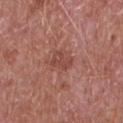| key | value |
|---|---|
| follow-up | imaged on a skin check; not biopsied |
| tile lighting | white-light |
| image | ~15 mm tile from a whole-body skin photo |
| anatomic site | the chest |
| patient | male, roughly 65 years of age |
| automated metrics | an area of roughly 4 mm², an eccentricity of roughly 0.7, and a symmetry-axis asymmetry near 0.3; a mean CIELAB color near L≈46 a*≈26 b*≈26 and a lesion–skin lightness drop of about 8; a border-irregularity index near 3.5/10, a color-variation rating of about 2/10, and a peripheral color-asymmetry measure near 1 |